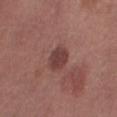Imaged during a routine full-body skin examination; the lesion was not biopsied and no histopathology is available.